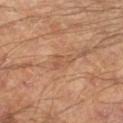Recorded during total-body skin imaging; not selected for excision or biopsy. About 3 mm across. Located on the right lower leg. The lesion-visualizer software estimated a footprint of about 4.5 mm² and an eccentricity of roughly 0.8. The software also gave an average lesion color of about L≈53 a*≈21 b*≈32 (CIELAB), a lesion–skin lightness drop of about 7, and a lesion-to-skin contrast of about 5 (normalized; higher = more distinct). A male subject approximately 65 years of age. Cropped from a whole-body photographic skin survey; the tile spans about 15 mm.